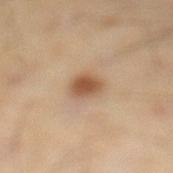workup = imaged on a skin check; not biopsied | image source = ~15 mm tile from a whole-body skin photo | patient = male, about 45 years old | site = the leg | tile lighting = cross-polarized.